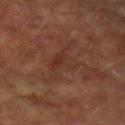Assessment:
Part of a total-body skin-imaging series; this lesion was reviewed on a skin check and was not flagged for biopsy.
Background:
A male patient in their mid- to late 70s. The lesion's longest dimension is about 6 mm. A close-up tile cropped from a whole-body skin photograph, about 15 mm across. The tile uses cross-polarized illumination. The lesion is located on the left forearm.